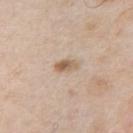Imaged during a routine full-body skin examination; the lesion was not biopsied and no histopathology is available. This image is a 15 mm lesion crop taken from a total-body photograph. On the left upper arm. This is a white-light tile. Approximately 2.5 mm at its widest. The total-body-photography lesion software estimated border irregularity of about 3 on a 0–10 scale and internal color variation of about 6 on a 0–10 scale. A male patient approximately 55 years of age.